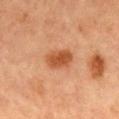workup=imaged on a skin check; not biopsied | illumination=cross-polarized | size=≈3.5 mm | location=the chest | subject=female, aged 58 to 62 | automated lesion analysis=a shape eccentricity near 0.75; border irregularity of about 1.5 on a 0–10 scale and peripheral color asymmetry of about 1; a nevus-likeness score of about 95/100 and a lesion-detection confidence of about 100/100 | acquisition=total-body-photography crop, ~15 mm field of view.